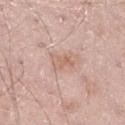Part of a total-body skin-imaging series; this lesion was reviewed on a skin check and was not flagged for biopsy.
The tile uses white-light illumination.
A male patient approximately 60 years of age.
A 15 mm close-up extracted from a 3D total-body photography capture.
Automated image analysis of the tile measured an area of roughly 4.5 mm² and two-axis asymmetry of about 0.3. The analysis additionally found border irregularity of about 3 on a 0–10 scale, internal color variation of about 2.5 on a 0–10 scale, and peripheral color asymmetry of about 1. It also reported an automated nevus-likeness rating near 0 out of 100 and a lesion-detection confidence of about 100/100.
The lesion is on the right lower leg.